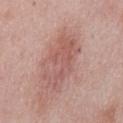Imaged during a routine full-body skin examination; the lesion was not biopsied and no histopathology is available. Cropped from a total-body skin-imaging series; the visible field is about 15 mm. The recorded lesion diameter is about 7 mm. From the abdomen. A male subject roughly 55 years of age.